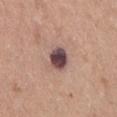follow-up = total-body-photography surveillance lesion; no biopsy
subject = female, about 45 years old
anatomic site = the abdomen
automated metrics = a border-irregularity rating of about 1.5/10 and radial color variation of about 2; an automated nevus-likeness rating near 10 out of 100 and a detector confidence of about 100 out of 100 that the crop contains a lesion
diameter = ≈3 mm
tile lighting = white-light illumination
image source = ~15 mm crop, total-body skin-cancer survey